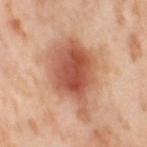- notes — no biopsy performed (imaged during a skin exam)
- subject — female, approximately 55 years of age
- image-analysis metrics — a footprint of about 30 mm², an outline eccentricity of about 0.75 (0 = round, 1 = elongated), and two-axis asymmetry of about 0.3; a detector confidence of about 100 out of 100 that the crop contains a lesion
- image source — 15 mm crop, total-body photography
- anatomic site — the right thigh